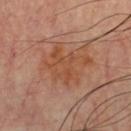Notes:
- anatomic site · the front of the torso
- patient · male, aged 48–52
- image source · ~15 mm crop, total-body skin-cancer survey
- illumination · cross-polarized illumination